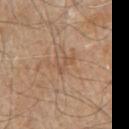No biopsy was performed on this lesion — it was imaged during a full skin examination and was not determined to be concerning. A lesion tile, about 15 mm wide, cut from a 3D total-body photograph. From the arm. A male patient aged 78–82. The recorded lesion diameter is about 2.5 mm.